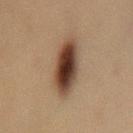Captured during whole-body skin photography for melanoma surveillance; the lesion was not biopsied.
Captured under cross-polarized illumination.
A 15 mm crop from a total-body photograph taken for skin-cancer surveillance.
A female subject, in their 60s.
The lesion is on the mid back.
Approximately 6 mm at its widest.